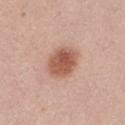Background: A female subject, aged around 20. Cropped from a total-body skin-imaging series; the visible field is about 15 mm. On the left thigh.